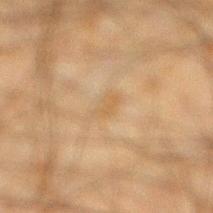Part of a total-body skin-imaging series; this lesion was reviewed on a skin check and was not flagged for biopsy.
Automated tile analysis of the lesion measured an average lesion color of about L≈47 a*≈13 b*≈31 (CIELAB) and a normalized lesion–skin contrast near 4.5. And it measured internal color variation of about 1.5 on a 0–10 scale and radial color variation of about 0.5.
Cropped from a total-body skin-imaging series; the visible field is about 15 mm.
Longest diameter approximately 3 mm.
Imaged with cross-polarized lighting.
On the right lower leg.
A male subject, aged 33–37.